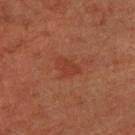notes: total-body-photography surveillance lesion; no biopsy | anatomic site: the left forearm | subject: female, aged approximately 65 | tile lighting: cross-polarized illumination | size: ≈3 mm | image source: ~15 mm tile from a whole-body skin photo.